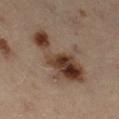Case summary:
- follow-up — catalogued during a skin exam; not biopsied
- acquisition — 15 mm crop, total-body photography
- anatomic site — the right thigh
- size — ≈8 mm
- patient — male, in their mid-50s
- TBP lesion metrics — a mean CIELAB color near L≈38 a*≈16 b*≈25, a lesion–skin lightness drop of about 13, and a normalized lesion–skin contrast near 11; an automated nevus-likeness rating near 70 out of 100 and a detector confidence of about 100 out of 100 that the crop contains a lesion
- lighting — cross-polarized